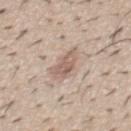<tbp_lesion>
<biopsy_status>not biopsied; imaged during a skin examination</biopsy_status>
<image>
  <source>total-body photography crop</source>
  <field_of_view_mm>15</field_of_view_mm>
</image>
<patient>
  <sex>male</sex>
  <age_approx>40</age_approx>
</patient>
<lesion_size>
  <long_diameter_mm_approx>4.0</long_diameter_mm_approx>
</lesion_size>
<lighting>white-light</lighting>
<site>abdomen</site>
</tbp_lesion>